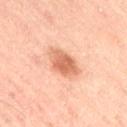Q: How was this image acquired?
A: total-body-photography crop, ~15 mm field of view
Q: Lesion location?
A: the leg
Q: Patient demographics?
A: male, aged 63 to 67